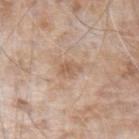Impression: Part of a total-body skin-imaging series; this lesion was reviewed on a skin check and was not flagged for biopsy. Acquisition and patient details: Longest diameter approximately 2.5 mm. The lesion-visualizer software estimated an average lesion color of about L≈59 a*≈18 b*≈30 (CIELAB), a lesion–skin lightness drop of about 8, and a normalized border contrast of about 5.5. And it measured a nevus-likeness score of about 0/100 and a lesion-detection confidence of about 100/100. A male patient, aged 73–77. This is a white-light tile. The lesion is located on the left forearm. A roughly 15 mm field-of-view crop from a total-body skin photograph.